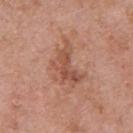Imaged during a routine full-body skin examination; the lesion was not biopsied and no histopathology is available. A roughly 15 mm field-of-view crop from a total-body skin photograph. On the chest. Measured at roughly 5.5 mm in maximum diameter. The tile uses white-light illumination. The patient is a male about 70 years old.